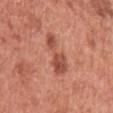follow-up = total-body-photography surveillance lesion; no biopsy
location = the left upper arm
tile lighting = white-light illumination
lesion size = ≈5 mm
imaging modality = 15 mm crop, total-body photography
patient = female, aged approximately 50